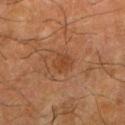Impression:
No biopsy was performed on this lesion — it was imaged during a full skin examination and was not determined to be concerning.
Acquisition and patient details:
About 3 mm across. This is a cross-polarized tile. The lesion is located on the left lower leg. The subject is a male approximately 65 years of age. The total-body-photography lesion software estimated an area of roughly 5 mm², an eccentricity of roughly 0.6, and a shape-asymmetry score of about 0.2 (0 = symmetric). The software also gave a classifier nevus-likeness of about 10/100 and lesion-presence confidence of about 100/100. Cropped from a whole-body photographic skin survey; the tile spans about 15 mm.